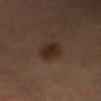Q: What did automated image analysis measure?
A: a mean CIELAB color near L≈20 a*≈15 b*≈19, a lesion–skin lightness drop of about 7, and a normalized lesion–skin contrast near 9; a border-irregularity index near 1.5/10 and peripheral color asymmetry of about 0.5; a lesion-detection confidence of about 100/100
Q: Patient demographics?
A: male, roughly 30 years of age
Q: What kind of image is this?
A: ~15 mm crop, total-body skin-cancer survey
Q: How was the tile lit?
A: cross-polarized
Q: What is the anatomic site?
A: the head or neck
Q: How large is the lesion?
A: ≈3 mm